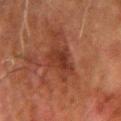No biopsy was performed on this lesion — it was imaged during a full skin examination and was not determined to be concerning. A roughly 15 mm field-of-view crop from a total-body skin photograph. On the right forearm. The patient is a male aged around 80.